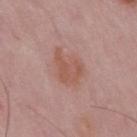biopsy status=imaged on a skin check; not biopsied | subject=male, approximately 50 years of age | automated lesion analysis=a footprint of about 11 mm², a shape eccentricity near 0.65, and two-axis asymmetry of about 0.25; a color-variation rating of about 2.5/10 and peripheral color asymmetry of about 1 | lighting=white-light | anatomic site=the mid back | diameter=≈4.5 mm | image source=~15 mm tile from a whole-body skin photo.